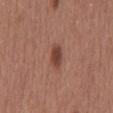Imaged during a routine full-body skin examination; the lesion was not biopsied and no histopathology is available.
The lesion's longest dimension is about 3 mm.
The lesion is located on the mid back.
This is a white-light tile.
A roughly 15 mm field-of-view crop from a total-body skin photograph.
A male patient in their mid-60s.